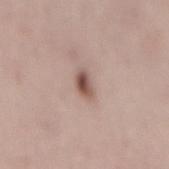notes — total-body-photography surveillance lesion; no biopsy
size — ≈2.5 mm
image — total-body-photography crop, ~15 mm field of view
lighting — white-light illumination
patient — female, aged around 50
site — the mid back